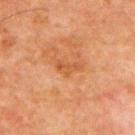| key | value |
|---|---|
| lesion size | ≈3 mm |
| illumination | cross-polarized |
| subject | male, in their 80s |
| location | the mid back |
| image source | ~15 mm tile from a whole-body skin photo |
| TBP lesion metrics | a lesion color around L≈43 a*≈22 b*≈34 in CIELAB and a lesion-to-skin contrast of about 5 (normalized; higher = more distinct) |